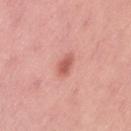Q: Was this lesion biopsied?
A: catalogued during a skin exam; not biopsied